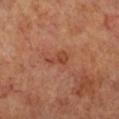Q: Illumination type?
A: cross-polarized illumination
Q: Where on the body is the lesion?
A: the leg
Q: What kind of image is this?
A: 15 mm crop, total-body photography
Q: Lesion size?
A: ≈3 mm
Q: What are the patient's age and sex?
A: female, aged 53 to 57